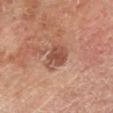{"image": {"source": "total-body photography crop", "field_of_view_mm": 15}, "lighting": "cross-polarized", "patient": {"sex": "male", "age_approx": 70}, "lesion_size": {"long_diameter_mm_approx": 4.0}, "site": "right lower leg"}